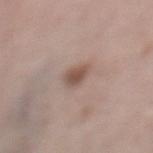This lesion was catalogued during total-body skin photography and was not selected for biopsy.
A female patient about 65 years old.
This is a white-light tile.
A 15 mm crop from a total-body photograph taken for skin-cancer surveillance.
The lesion is located on the leg.